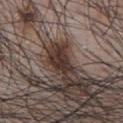Impression: Part of a total-body skin-imaging series; this lesion was reviewed on a skin check and was not flagged for biopsy. Context: Captured under white-light illumination. The lesion is on the chest. The recorded lesion diameter is about 3 mm. The patient is a male aged around 70. Automated tile analysis of the lesion measured an area of roughly 5 mm² and an eccentricity of roughly 0.75. It also reported a border-irregularity index near 4/10, a color-variation rating of about 2/10, and radial color variation of about 0.5. The software also gave a nevus-likeness score of about 65/100 and lesion-presence confidence of about 65/100. A 15 mm crop from a total-body photograph taken for skin-cancer surveillance.